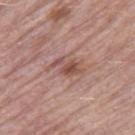Q: Is there a histopathology result?
A: imaged on a skin check; not biopsied
Q: What kind of image is this?
A: 15 mm crop, total-body photography
Q: How was the tile lit?
A: white-light
Q: What are the patient's age and sex?
A: female, aged 68–72
Q: Where on the body is the lesion?
A: the right thigh
Q: How large is the lesion?
A: about 4.5 mm